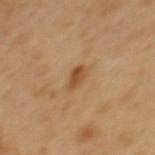Q: Is there a histopathology result?
A: imaged on a skin check; not biopsied